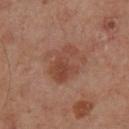workup = total-body-photography surveillance lesion; no biopsy
patient = male, approximately 50 years of age
location = the left lower leg
imaging modality = ~15 mm crop, total-body skin-cancer survey
illumination = cross-polarized illumination